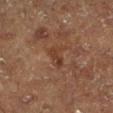Q: Was a biopsy performed?
A: total-body-photography surveillance lesion; no biopsy
Q: Who is the patient?
A: male, roughly 75 years of age
Q: What did automated image analysis measure?
A: a lesion area of about 4 mm², a shape eccentricity near 0.85, and two-axis asymmetry of about 0.4; a nevus-likeness score of about 0/100
Q: What kind of image is this?
A: ~15 mm crop, total-body skin-cancer survey
Q: Lesion location?
A: the left lower leg
Q: What is the lesion's diameter?
A: about 3 mm
Q: How was the tile lit?
A: cross-polarized illumination